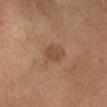follow-up: catalogued during a skin exam; not biopsied
image source: ~15 mm crop, total-body skin-cancer survey
lighting: cross-polarized illumination
patient: female, in their 60s
location: the head or neck